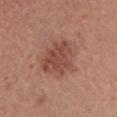Case summary:
– biopsy status — imaged on a skin check; not biopsied